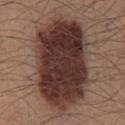Clinical impression:
Captured during whole-body skin photography for melanoma surveillance; the lesion was not biopsied.
Context:
The tile uses white-light illumination. The total-body-photography lesion software estimated an automated nevus-likeness rating near 15 out of 100. A region of skin cropped from a whole-body photographic capture, roughly 15 mm wide. A male patient in their 40s. Approximately 12.5 mm at its widest. Located on the mid back.